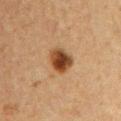Notes:
- patient · female, aged around 40
- diameter · ≈3.5 mm
- tile lighting · cross-polarized illumination
- image source · ~15 mm tile from a whole-body skin photo
- automated metrics · a border-irregularity index near 1.5/10, a within-lesion color-variation index near 5.5/10, and radial color variation of about 1.5; a classifier nevus-likeness of about 100/100 and a lesion-detection confidence of about 100/100
- anatomic site · the chest